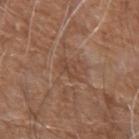Q: Is there a histopathology result?
A: no biopsy performed (imaged during a skin exam)
Q: What did automated image analysis measure?
A: a lesion area of about 2.5 mm², an outline eccentricity of about 0.9 (0 = round, 1 = elongated), and a shape-asymmetry score of about 0.4 (0 = symmetric); roughly 6 lightness units darker than nearby skin and a normalized lesion–skin contrast near 5; an automated nevus-likeness rating near 0 out of 100
Q: How was the tile lit?
A: white-light illumination
Q: What is the anatomic site?
A: the left forearm
Q: What is the lesion's diameter?
A: ≈2.5 mm
Q: What kind of image is this?
A: 15 mm crop, total-body photography
Q: Patient demographics?
A: male, roughly 80 years of age